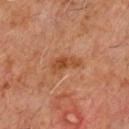Case summary:
• notes — total-body-photography surveillance lesion; no biopsy
• patient — male, about 60 years old
• imaging modality — 15 mm crop, total-body photography
• site — the chest